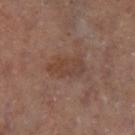<case>
  <biopsy_status>not biopsied; imaged during a skin examination</biopsy_status>
  <patient>
    <sex>female</sex>
    <age_approx>60</age_approx>
  </patient>
  <site>left lower leg</site>
  <image>
    <source>total-body photography crop</source>
    <field_of_view_mm>15</field_of_view_mm>
  </image>
  <automated_metrics>
    <shape_asymmetry>0.2</shape_asymmetry>
    <lesion_detection_confidence_0_100>100</lesion_detection_confidence_0_100>
  </automated_metrics>
  <lighting>cross-polarized</lighting>
</case>